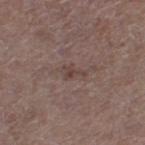follow-up = catalogued during a skin exam; not biopsied
site = the left thigh
subject = female, aged 48 to 52
imaging modality = ~15 mm crop, total-body skin-cancer survey
diameter = about 2.5 mm
tile lighting = white-light illumination
image-analysis metrics = a footprint of about 3 mm² and two-axis asymmetry of about 0.5